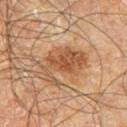  biopsy_status: not biopsied; imaged during a skin examination
  lesion_size:
    long_diameter_mm_approx: 6.0
  lighting: cross-polarized
  automated_metrics:
    area_mm2_approx: 15.0
    eccentricity: 0.8
    shape_asymmetry: 0.4
    color_variation_0_10: 3.5
    peripheral_color_asymmetry: 1.0
  patient:
    sex: male
    age_approx: 60
  site: right leg
  image:
    source: total-body photography crop
    field_of_view_mm: 15